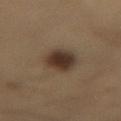Clinical impression: The lesion was photographed on a routine skin check and not biopsied; there is no pathology result. Acquisition and patient details: The total-body-photography lesion software estimated an area of roughly 9.5 mm², a shape eccentricity near 0.75, and two-axis asymmetry of about 0.25. The analysis additionally found a border-irregularity index near 2.5/10, internal color variation of about 4.5 on a 0–10 scale, and radial color variation of about 1.5. The lesion is located on the lower back. A roughly 15 mm field-of-view crop from a total-body skin photograph. Imaged with cross-polarized lighting. The recorded lesion diameter is about 4.5 mm. A male patient, roughly 60 years of age.